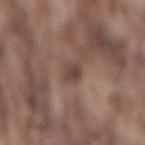  biopsy_status: not biopsied; imaged during a skin examination
  image:
    source: total-body photography crop
    field_of_view_mm: 15
  lighting: white-light
  lesion_size:
    long_diameter_mm_approx: 3.0
  automated_metrics:
    area_mm2_approx: 4.0
    eccentricity: 0.8
    shape_asymmetry: 0.2
    border_irregularity_0_10: 2.0
    color_variation_0_10: 2.5
    peripheral_color_asymmetry: 1.0
    nevus_likeness_0_100: 0
  site: back
  patient:
    sex: male
    age_approx: 75